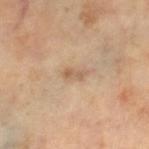Impression:
Imaged during a routine full-body skin examination; the lesion was not biopsied and no histopathology is available.
Image and clinical context:
Cropped from a whole-body photographic skin survey; the tile spans about 15 mm. The lesion is located on the right lower leg. This is a cross-polarized tile. A patient aged approximately 60. Approximately 2.5 mm at its widest.